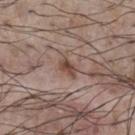<lesion>
<biopsy_status>not biopsied; imaged during a skin examination</biopsy_status>
<patient>
  <sex>male</sex>
  <age_approx>75</age_approx>
</patient>
<image>
  <source>total-body photography crop</source>
  <field_of_view_mm>15</field_of_view_mm>
</image>
<lighting>white-light</lighting>
<automated_metrics>
  <shape_asymmetry>0.35</shape_asymmetry>
  <border_irregularity_0_10>3.0</border_irregularity_0_10>
  <peripheral_color_asymmetry>1.5</peripheral_color_asymmetry>
</automated_metrics>
<site>chest</site>
</lesion>